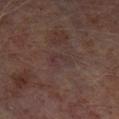Q: Was this lesion biopsied?
A: total-body-photography surveillance lesion; no biopsy
Q: What are the patient's age and sex?
A: male, roughly 65 years of age
Q: What is the anatomic site?
A: the left lower leg
Q: What kind of image is this?
A: 15 mm crop, total-body photography